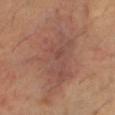biopsy_status: not biopsied; imaged during a skin examination
image:
  source: total-body photography crop
  field_of_view_mm: 15
site: right thigh
patient:
  sex: male
  age_approx: 60